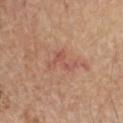Imaged during a routine full-body skin examination; the lesion was not biopsied and no histopathology is available. A 15 mm crop from a total-body photograph taken for skin-cancer surveillance. Automated image analysis of the tile measured a within-lesion color-variation index near 1/10. The analysis additionally found a classifier nevus-likeness of about 0/100 and a lesion-detection confidence of about 100/100. Measured at roughly 4 mm in maximum diameter. Located on the arm. The subject is a female in their mid-60s. Captured under cross-polarized illumination.